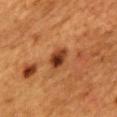Notes:
- notes: no biopsy performed (imaged during a skin exam)
- lesion diameter: ≈3 mm
- automated metrics: about 12 CIELAB-L* units darker than the surrounding skin and a normalized lesion–skin contrast near 11; a within-lesion color-variation index near 6.5/10 and a peripheral color-asymmetry measure near 2.5
- subject: female, aged around 55
- image source: total-body-photography crop, ~15 mm field of view
- lighting: cross-polarized illumination
- site: the mid back